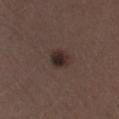Clinical impression:
The lesion was tiled from a total-body skin photograph and was not biopsied.
Image and clinical context:
An algorithmic analysis of the crop reported an area of roughly 5 mm², a shape eccentricity near 0.45, and two-axis asymmetry of about 0.2. And it measured a lesion–skin lightness drop of about 10 and a lesion-to-skin contrast of about 10.5 (normalized; higher = more distinct). Measured at roughly 3 mm in maximum diameter. From the right lower leg. Imaged with white-light lighting. A 15 mm close-up extracted from a 3D total-body photography capture. The patient is a male aged 48–52.